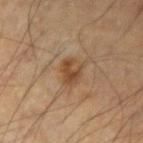biopsy status=no biopsy performed (imaged during a skin exam); subject=male, approximately 60 years of age; site=the left forearm; acquisition=~15 mm crop, total-body skin-cancer survey.